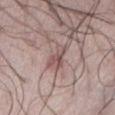<case>
<biopsy_status>not biopsied; imaged during a skin examination</biopsy_status>
<image>
  <source>total-body photography crop</source>
  <field_of_view_mm>15</field_of_view_mm>
</image>
<automated_metrics>
  <area_mm2_approx>5.5</area_mm2_approx>
  <color_variation_0_10>4.0</color_variation_0_10>
  <nevus_likeness_0_100>0</nevus_likeness_0_100>
  <lesion_detection_confidence_0_100>80</lesion_detection_confidence_0_100>
</automated_metrics>
<lesion_size>
  <long_diameter_mm_approx>3.5</long_diameter_mm_approx>
</lesion_size>
<site>abdomen</site>
<patient>
  <sex>male</sex>
  <age_approx>25</age_approx>
</patient>
</case>